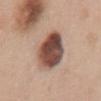follow-up = catalogued during a skin exam; not biopsied | tile lighting = white-light | acquisition = total-body-photography crop, ~15 mm field of view | patient = female, in their 50s | site = the back | lesion diameter = ≈5.5 mm.